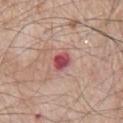patient:
  sex: male
  age_approx: 80
image:
  source: total-body photography crop
  field_of_view_mm: 15
lesion_size:
  long_diameter_mm_approx: 3.0
lighting: white-light
site: chest
automated_metrics:
  area_mm2_approx: 4.5
  eccentricity: 0.65
  shape_asymmetry: 0.25
  cielab_L: 50
  cielab_a: 32
  cielab_b: 22
  vs_skin_darker_L: 14.0
  vs_skin_contrast_norm: 10.0
  border_irregularity_0_10: 2.0
  peripheral_color_asymmetry: 1.0